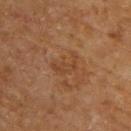patient = male, roughly 65 years of age; body site = the upper back; image = total-body-photography crop, ~15 mm field of view.